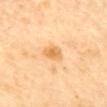Notes:
- follow-up: no biopsy performed (imaged during a skin exam)
- location: the mid back
- illumination: cross-polarized illumination
- lesion size: about 3 mm
- TBP lesion metrics: a border-irregularity index near 2/10, internal color variation of about 3.5 on a 0–10 scale, and peripheral color asymmetry of about 1
- acquisition: total-body-photography crop, ~15 mm field of view
- patient: female, aged 58 to 62